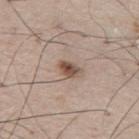| feature | finding |
|---|---|
| notes | total-body-photography surveillance lesion; no biopsy |